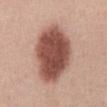Captured during whole-body skin photography for melanoma surveillance; the lesion was not biopsied.
Approximately 7.5 mm at its widest.
Imaged with white-light lighting.
The subject is a male approximately 55 years of age.
Cropped from a total-body skin-imaging series; the visible field is about 15 mm.
On the abdomen.